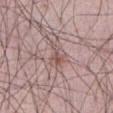Q: Is there a histopathology result?
A: imaged on a skin check; not biopsied
Q: Who is the patient?
A: male, approximately 65 years of age
Q: What is the anatomic site?
A: the front of the torso
Q: How was this image acquired?
A: ~15 mm crop, total-body skin-cancer survey
Q: Lesion size?
A: ~3 mm (longest diameter)
Q: Illumination type?
A: white-light